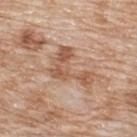| field | value |
|---|---|
| biopsy status | imaged on a skin check; not biopsied |
| subject | male, aged 78 to 82 |
| body site | the upper back |
| imaging modality | 15 mm crop, total-body photography |